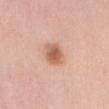Notes:
• biopsy status · catalogued during a skin exam; not biopsied
• patient · female, aged around 50
• anatomic site · the lower back
• imaging modality · total-body-photography crop, ~15 mm field of view